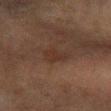Notes:
- biopsy status · catalogued during a skin exam; not biopsied